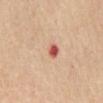Clinical impression: Recorded during total-body skin imaging; not selected for excision or biopsy.